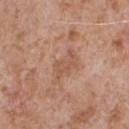The lesion was photographed on a routine skin check and not biopsied; there is no pathology result.